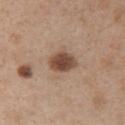Case summary:
– biopsy status — total-body-photography surveillance lesion; no biopsy
– body site — the arm
– imaging modality — total-body-photography crop, ~15 mm field of view
– patient — female, aged approximately 40
– illumination — white-light illumination
– lesion size — ~3 mm (longest diameter)